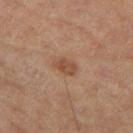The lesion was tiled from a total-body skin photograph and was not biopsied. Automated tile analysis of the lesion measured border irregularity of about 2 on a 0–10 scale, a within-lesion color-variation index near 2.5/10, and radial color variation of about 1. A male subject, in their mid-80s or older. The lesion is located on the leg. A 15 mm crop from a total-body photograph taken for skin-cancer surveillance. About 3 mm across. Captured under cross-polarized illumination.